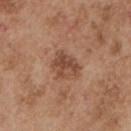Recorded during total-body skin imaging; not selected for excision or biopsy. The recorded lesion diameter is about 4 mm. A close-up tile cropped from a whole-body skin photograph, about 15 mm across. Imaged with white-light lighting. A male subject, aged 53 to 57. From the chest.